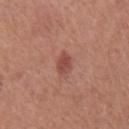biopsy_status: not biopsied; imaged during a skin examination
patient:
  sex: male
  age_approx: 65
site: chest
automated_metrics:
  vs_skin_darker_L: 9.0
  vs_skin_contrast_norm: 7.0
  border_irregularity_0_10: 1.5
  color_variation_0_10: 2.5
  peripheral_color_asymmetry: 1.0
lighting: white-light
image:
  source: total-body photography crop
  field_of_view_mm: 15